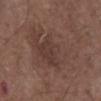workup = total-body-photography surveillance lesion; no biopsy
diameter = ≈8.5 mm
subject = male, aged approximately 70
tile lighting = white-light illumination
body site = the front of the torso
imaging modality = ~15 mm crop, total-body skin-cancer survey
image-analysis metrics = an average lesion color of about L≈40 a*≈17 b*≈22 (CIELAB) and about 5 CIELAB-L* units darker than the surrounding skin; border irregularity of about 7.5 on a 0–10 scale; a classifier nevus-likeness of about 5/100